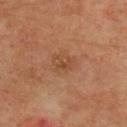| field | value |
|---|---|
| biopsy status | catalogued during a skin exam; not biopsied |
| patient | male, roughly 65 years of age |
| lesion diameter | ≈3 mm |
| image | ~15 mm tile from a whole-body skin photo |
| location | the upper back |
| automated lesion analysis | an eccentricity of roughly 0.7; about 6 CIELAB-L* units darker than the surrounding skin and a normalized border contrast of about 5; a border-irregularity rating of about 3/10, a color-variation rating of about 3/10, and peripheral color asymmetry of about 1; an automated nevus-likeness rating near 0 out of 100 |
| lighting | cross-polarized |